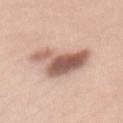Part of a total-body skin-imaging series; this lesion was reviewed on a skin check and was not flagged for biopsy.
Located on the mid back.
This is a white-light tile.
Measured at roughly 6 mm in maximum diameter.
A female patient aged 33 to 37.
A region of skin cropped from a whole-body photographic capture, roughly 15 mm wide.